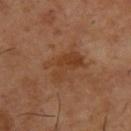This lesion was catalogued during total-body skin photography and was not selected for biopsy.
This is a cross-polarized tile.
About 4.5 mm across.
The subject is a male aged around 55.
A roughly 15 mm field-of-view crop from a total-body skin photograph.
The lesion is on the back.
An algorithmic analysis of the crop reported a footprint of about 9 mm² and a symmetry-axis asymmetry near 0.4. And it measured a color-variation rating of about 3.5/10 and peripheral color asymmetry of about 1.5.